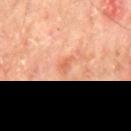Q: Is there a histopathology result?
A: imaged on a skin check; not biopsied
Q: Lesion location?
A: the mid back
Q: What are the patient's age and sex?
A: male, in their mid- to late 60s
Q: What did automated image analysis measure?
A: a mean CIELAB color near L≈63 a*≈29 b*≈36 and a normalized border contrast of about 5
Q: Lesion size?
A: ~2.5 mm (longest diameter)
Q: What kind of image is this?
A: ~15 mm crop, total-body skin-cancer survey